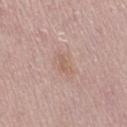Image and clinical context: The lesion is located on the leg. A region of skin cropped from a whole-body photographic capture, roughly 15 mm wide. A female patient aged 48–52.